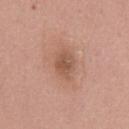The lesion was photographed on a routine skin check and not biopsied; there is no pathology result. The lesion is located on the chest. Approximately 3.5 mm at its widest. The patient is a male aged 53–57. A 15 mm close-up extracted from a 3D total-body photography capture. Imaged with white-light lighting.